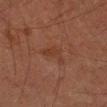{"biopsy_status": "not biopsied; imaged during a skin examination", "image": {"source": "total-body photography crop", "field_of_view_mm": 15}, "site": "arm", "lesion_size": {"long_diameter_mm_approx": 3.5}, "automated_metrics": {"cielab_L": 29, "cielab_a": 18, "cielab_b": 24, "vs_skin_darker_L": 4.0, "vs_skin_contrast_norm": 5.0, "color_variation_0_10": 1.5, "peripheral_color_asymmetry": 0.5, "nevus_likeness_0_100": 0, "lesion_detection_confidence_0_100": 100}, "lighting": "cross-polarized", "patient": {"sex": "male", "age_approx": 65}}